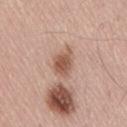Context: Cropped from a whole-body photographic skin survey; the tile spans about 15 mm. A male subject about 55 years old. Imaged with white-light lighting. The lesion is on the lower back.